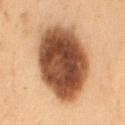  biopsy_status: not biopsied; imaged during a skin examination
  lighting: cross-polarized
  lesion_size:
    long_diameter_mm_approx: 9.0
  image:
    source: total-body photography crop
    field_of_view_mm: 15
  patient:
    sex: male
    age_approx: 55
  automated_metrics:
    vs_skin_contrast_norm: 15.5
    border_irregularity_0_10: 1.5
    color_variation_0_10: 6.5
    peripheral_color_asymmetry: 2.0
    nevus_likeness_0_100: 100
    lesion_detection_confidence_0_100: 100
  site: back